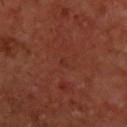notes=total-body-photography surveillance lesion; no biopsy | patient=male, in their 60s | lighting=cross-polarized | lesion diameter=≈1 mm | site=the upper back | acquisition=~15 mm tile from a whole-body skin photo.